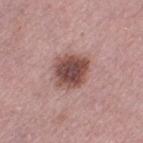* notes — imaged on a skin check; not biopsied
* acquisition — total-body-photography crop, ~15 mm field of view
* diameter — about 4 mm
* lighting — white-light illumination
* anatomic site — the left thigh
* patient — female, roughly 70 years of age